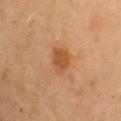Assessment:
The lesion was tiled from a total-body skin photograph and was not biopsied.
Context:
This image is a 15 mm lesion crop taken from a total-body photograph. The subject is a female roughly 70 years of age. The lesion is located on the left upper arm.